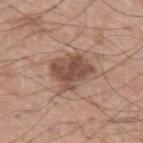{
  "biopsy_status": "not biopsied; imaged during a skin examination",
  "image": {
    "source": "total-body photography crop",
    "field_of_view_mm": 15
  },
  "automated_metrics": {
    "vs_skin_contrast_norm": 8.5,
    "border_irregularity_0_10": 2.5,
    "color_variation_0_10": 4.0,
    "peripheral_color_asymmetry": 1.5,
    "nevus_likeness_0_100": 60,
    "lesion_detection_confidence_0_100": 100
  },
  "patient": {
    "sex": "male",
    "age_approx": 55
  },
  "lesion_size": {
    "long_diameter_mm_approx": 4.5
  },
  "lighting": "white-light"
}